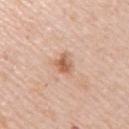notes — total-body-photography surveillance lesion; no biopsy
subject — female, aged 43 to 47
image source — ~15 mm tile from a whole-body skin photo
TBP lesion metrics — a border-irregularity index near 3.5/10, a within-lesion color-variation index near 4.5/10, and radial color variation of about 1.5; an automated nevus-likeness rating near 85 out of 100
lighting — white-light
body site — the right upper arm